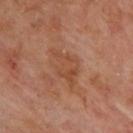{
  "biopsy_status": "not biopsied; imaged during a skin examination",
  "patient": {
    "sex": "male",
    "age_approx": 70
  },
  "automated_metrics": {
    "cielab_L": 46,
    "cielab_a": 23,
    "cielab_b": 32,
    "vs_skin_darker_L": 6.0,
    "vs_skin_contrast_norm": 5.0,
    "border_irregularity_0_10": 5.0,
    "lesion_detection_confidence_0_100": 100
  },
  "lesion_size": {
    "long_diameter_mm_approx": 5.0
  },
  "image": {
    "source": "total-body photography crop",
    "field_of_view_mm": 15
  },
  "site": "upper back",
  "lighting": "cross-polarized"
}